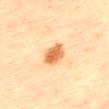{"lesion_size": {"long_diameter_mm_approx": 4.5}, "image": {"source": "total-body photography crop", "field_of_view_mm": 15}, "patient": {"sex": "female", "age_approx": 65}, "site": "mid back"}